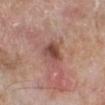Notes:
* workup — catalogued during a skin exam; not biopsied
* subject — male, in their 80s
* image source — 15 mm crop, total-body photography
* tile lighting — white-light illumination
* body site — the left lower leg